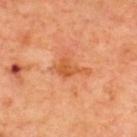Part of a total-body skin-imaging series; this lesion was reviewed on a skin check and was not flagged for biopsy. The lesion-visualizer software estimated an average lesion color of about L≈57 a*≈31 b*≈43 (CIELAB), roughly 9 lightness units darker than nearby skin, and a normalized border contrast of about 6.5. And it measured a lesion-detection confidence of about 100/100. The subject is in their mid- to late 60s. Approximately 4 mm at its widest. A region of skin cropped from a whole-body photographic capture, roughly 15 mm wide. The lesion is on the back.